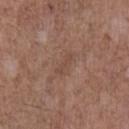* workup: catalogued during a skin exam; not biopsied